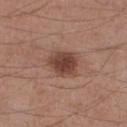workup: no biopsy performed (imaged during a skin exam)